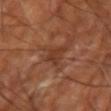Background:
This image is a 15 mm lesion crop taken from a total-body photograph. From the right upper arm. Automated image analysis of the tile measured a shape eccentricity near 0.55 and a shape-asymmetry score of about 0.3 (0 = symmetric). It also reported an average lesion color of about L≈36 a*≈23 b*≈30 (CIELAB) and a normalized lesion–skin contrast near 6. The software also gave border irregularity of about 3 on a 0–10 scale and internal color variation of about 2.5 on a 0–10 scale. About 3 mm across. The subject is about 65 years old. Imaged with cross-polarized lighting.